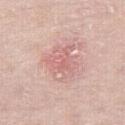Part of a total-body skin-imaging series; this lesion was reviewed on a skin check and was not flagged for biopsy. The patient is a female in their 70s. A roughly 15 mm field-of-view crop from a total-body skin photograph. The lesion is located on the right thigh.